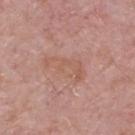Clinical impression:
The lesion was photographed on a routine skin check and not biopsied; there is no pathology result.
Acquisition and patient details:
The lesion-visualizer software estimated a lesion area of about 9.5 mm² and an eccentricity of roughly 0.9. The analysis additionally found an average lesion color of about L≈57 a*≈21 b*≈28 (CIELAB), about 5 CIELAB-L* units darker than the surrounding skin, and a normalized border contrast of about 5. And it measured a border-irregularity index near 5/10, a within-lesion color-variation index near 2.5/10, and peripheral color asymmetry of about 0.5. The software also gave a classifier nevus-likeness of about 0/100 and a lesion-detection confidence of about 100/100. Located on the upper back. Captured under white-light illumination. A male patient in their mid- to late 60s. Approximately 5 mm at its widest. A 15 mm crop from a total-body photograph taken for skin-cancer surveillance.